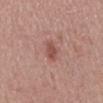Findings:
* workup · imaged on a skin check; not biopsied
* tile lighting · white-light
* imaging modality · total-body-photography crop, ~15 mm field of view
* subject · male, approximately 60 years of age
* location · the mid back